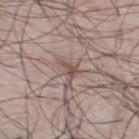{
  "biopsy_status": "not biopsied; imaged during a skin examination",
  "automated_metrics": {
    "area_mm2_approx": 3.0,
    "eccentricity": 0.8,
    "cielab_L": 51,
    "cielab_a": 16,
    "cielab_b": 22,
    "vs_skin_darker_L": 9.0,
    "border_irregularity_0_10": 5.0,
    "color_variation_0_10": 2.0,
    "peripheral_color_asymmetry": 0.5
  },
  "lighting": "white-light",
  "image": {
    "source": "total-body photography crop",
    "field_of_view_mm": 15
  },
  "site": "mid back",
  "patient": {
    "sex": "male",
    "age_approx": 45
  }
}